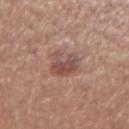{
  "biopsy_status": "not biopsied; imaged during a skin examination",
  "site": "left forearm",
  "lighting": "white-light",
  "patient": {
    "sex": "female",
    "age_approx": 45
  },
  "image": {
    "source": "total-body photography crop",
    "field_of_view_mm": 15
  }
}